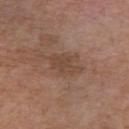Imaged during a routine full-body skin examination; the lesion was not biopsied and no histopathology is available. A roughly 15 mm field-of-view crop from a total-body skin photograph. Located on the front of the torso. A male patient, in their mid- to late 70s.